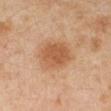follow-up = imaged on a skin check; not biopsied | illumination = cross-polarized illumination | body site = the left forearm | patient = female, roughly 40 years of age | image source = total-body-photography crop, ~15 mm field of view.